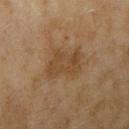notes: total-body-photography surveillance lesion; no biopsy | body site: the right upper arm | lighting: cross-polarized | automated lesion analysis: a mean CIELAB color near L≈40 a*≈16 b*≈31, about 7 CIELAB-L* units darker than the surrounding skin, and a normalized border contrast of about 6; a border-irregularity rating of about 3.5/10, a color-variation rating of about 2.5/10, and a peripheral color-asymmetry measure near 1 | size: about 4.5 mm | patient: female, aged approximately 60 | acquisition: 15 mm crop, total-body photography.